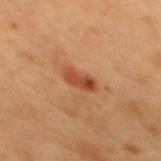biopsy_status: not biopsied; imaged during a skin examination
image:
  source: total-body photography crop
  field_of_view_mm: 15
patient:
  sex: female
  age_approx: 40
site: mid back